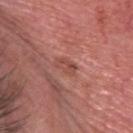Captured during whole-body skin photography for melanoma surveillance; the lesion was not biopsied.
The subject is a male aged around 65.
The lesion is on the head or neck.
Approximately 2.5 mm at its widest.
A 15 mm close-up tile from a total-body photography series done for melanoma screening.